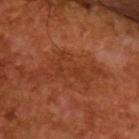Recorded during total-body skin imaging; not selected for excision or biopsy. A region of skin cropped from a whole-body photographic capture, roughly 15 mm wide. Captured under cross-polarized illumination. The patient is a male aged 63–67. The recorded lesion diameter is about 4.5 mm.